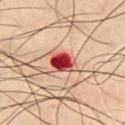Part of a total-body skin-imaging series; this lesion was reviewed on a skin check and was not flagged for biopsy. Captured under cross-polarized illumination. Longest diameter approximately 3 mm. The lesion is located on the chest. A male subject roughly 50 years of age. A 15 mm crop from a total-body photograph taken for skin-cancer surveillance.